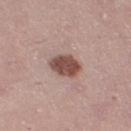Case summary:
- notes — total-body-photography surveillance lesion; no biopsy
- tile lighting — white-light illumination
- image — 15 mm crop, total-body photography
- subject — female, aged around 40
- lesion diameter — ~4 mm (longest diameter)
- body site — the right thigh
- image-analysis metrics — a lesion area of about 8.5 mm² and a shape eccentricity near 0.65; a mean CIELAB color near L≈49 a*≈21 b*≈23, about 15 CIELAB-L* units darker than the surrounding skin, and a lesion-to-skin contrast of about 10.5 (normalized; higher = more distinct); border irregularity of about 1 on a 0–10 scale and peripheral color asymmetry of about 1; a lesion-detection confidence of about 100/100